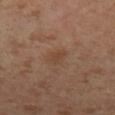Clinical impression:
This lesion was catalogued during total-body skin photography and was not selected for biopsy.
Clinical summary:
From the left lower leg. The tile uses cross-polarized illumination. A male subject, in their mid- to late 50s. The recorded lesion diameter is about 2.5 mm. A close-up tile cropped from a whole-body skin photograph, about 15 mm across.